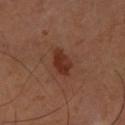{"biopsy_status": "not biopsied; imaged during a skin examination", "site": "right forearm", "patient": {"sex": "male", "age_approx": 60}, "image": {"source": "total-body photography crop", "field_of_view_mm": 15}, "automated_metrics": {"vs_skin_darker_L": 9.0}, "lighting": "cross-polarized"}